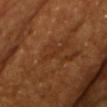biopsy status: no biopsy performed (imaged during a skin exam)
body site: the chest
image-analysis metrics: a lesion area of about 2.5 mm², an outline eccentricity of about 0.95 (0 = round, 1 = elongated), and a symmetry-axis asymmetry near 0.4; an average lesion color of about L≈32 a*≈23 b*≈32 (CIELAB), a lesion–skin lightness drop of about 5, and a lesion-to-skin contrast of about 5 (normalized; higher = more distinct)
size: ≈3.5 mm
patient: male, roughly 65 years of age
imaging modality: ~15 mm crop, total-body skin-cancer survey